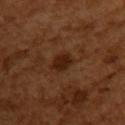workup: imaged on a skin check; not biopsied | lighting: cross-polarized | size: ~3 mm (longest diameter) | image-analysis metrics: a footprint of about 4.5 mm² and two-axis asymmetry of about 0.15; an average lesion color of about L≈23 a*≈21 b*≈28 (CIELAB), a lesion–skin lightness drop of about 9, and a normalized lesion–skin contrast near 9.5; a border-irregularity rating of about 1.5/10 and a within-lesion color-variation index near 1.5/10; a classifier nevus-likeness of about 70/100 and a detector confidence of about 100 out of 100 that the crop contains a lesion | location: the back | patient: female, about 55 years old | acquisition: total-body-photography crop, ~15 mm field of view.